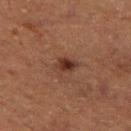From the left thigh. The total-body-photography lesion software estimated a symmetry-axis asymmetry near 0.25. And it measured a border-irregularity index near 2.5/10, internal color variation of about 5.5 on a 0–10 scale, and radial color variation of about 2. The subject is a male aged 73–77. This is a cross-polarized tile. Cropped from a whole-body photographic skin survey; the tile spans about 15 mm.